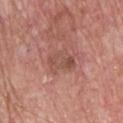| feature | finding |
|---|---|
| biopsy status | imaged on a skin check; not biopsied |
| subject | male, about 65 years old |
| imaging modality | ~15 mm tile from a whole-body skin photo |
| body site | the front of the torso |